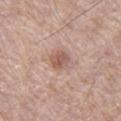{"biopsy_status": "not biopsied; imaged during a skin examination", "image": {"source": "total-body photography crop", "field_of_view_mm": 15}, "lesion_size": {"long_diameter_mm_approx": 2.5}, "patient": {"sex": "male", "age_approx": 70}, "site": "right thigh", "lighting": "white-light"}